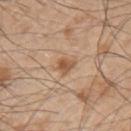anatomic site=the left upper arm | acquisition=total-body-photography crop, ~15 mm field of view | TBP lesion metrics=a lesion area of about 4 mm², a shape eccentricity near 0.7, and a symmetry-axis asymmetry near 0.3; a classifier nevus-likeness of about 70/100 and a detector confidence of about 100 out of 100 that the crop contains a lesion | lesion diameter=≈2.5 mm | patient=male, approximately 50 years of age | lighting=white-light illumination.